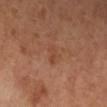follow-up — catalogued during a skin exam; not biopsied | image — 15 mm crop, total-body photography | body site — the leg | patient — female, aged around 65.